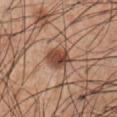No biopsy was performed on this lesion — it was imaged during a full skin examination and was not determined to be concerning.
This is a white-light tile.
A male patient about 55 years old.
Located on the abdomen.
Longest diameter approximately 3.5 mm.
A close-up tile cropped from a whole-body skin photograph, about 15 mm across.